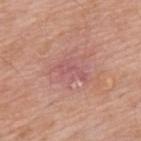{
  "biopsy_status": "not biopsied; imaged during a skin examination",
  "lighting": "white-light",
  "site": "back",
  "patient": {
    "sex": "male",
    "age_approx": 60
  },
  "image": {
    "source": "total-body photography crop",
    "field_of_view_mm": 15
  },
  "automated_metrics": {
    "area_mm2_approx": 4.5,
    "eccentricity": 0.9,
    "shape_asymmetry": 0.5,
    "cielab_L": 55,
    "cielab_a": 26,
    "cielab_b": 22,
    "vs_skin_darker_L": 6.0,
    "vs_skin_contrast_norm": 5.0,
    "nevus_likeness_0_100": 0,
    "lesion_detection_confidence_0_100": 95
  }
}